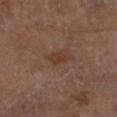The lesion was photographed on a routine skin check and not biopsied; there is no pathology result. Captured under cross-polarized illumination. The lesion is on the left lower leg. Longest diameter approximately 3 mm. A male subject approximately 70 years of age. An algorithmic analysis of the crop reported a footprint of about 3.5 mm², a shape eccentricity near 0.8, and a shape-asymmetry score of about 0.3 (0 = symmetric). The analysis additionally found roughly 6 lightness units darker than nearby skin and a normalized border contrast of about 6.5. The analysis additionally found a border-irregularity rating of about 3/10, internal color variation of about 2.5 on a 0–10 scale, and peripheral color asymmetry of about 1. The analysis additionally found an automated nevus-likeness rating near 0 out of 100 and a detector confidence of about 100 out of 100 that the crop contains a lesion. A region of skin cropped from a whole-body photographic capture, roughly 15 mm wide.